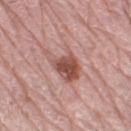follow-up: imaged on a skin check; not biopsied
lesion size: about 5 mm
TBP lesion metrics: an area of roughly 10 mm², an outline eccentricity of about 0.8 (0 = round, 1 = elongated), and two-axis asymmetry of about 0.4; a border-irregularity index near 5/10, a color-variation rating of about 5/10, and peripheral color asymmetry of about 1.5; a detector confidence of about 100 out of 100 that the crop contains a lesion
subject: female, about 65 years old
body site: the left leg
tile lighting: white-light
imaging modality: total-body-photography crop, ~15 mm field of view